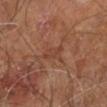Impression: Captured during whole-body skin photography for melanoma surveillance; the lesion was not biopsied. Context: Captured under cross-polarized illumination. Longest diameter approximately 3 mm. A male patient aged 58–62. The lesion-visualizer software estimated a lesion area of about 3.5 mm², an outline eccentricity of about 0.85 (0 = round, 1 = elongated), and a shape-asymmetry score of about 0.35 (0 = symmetric). The software also gave a color-variation rating of about 1/10 and peripheral color asymmetry of about 0. The software also gave an automated nevus-likeness rating near 0 out of 100 and lesion-presence confidence of about 90/100. Located on the right leg. A region of skin cropped from a whole-body photographic capture, roughly 15 mm wide.